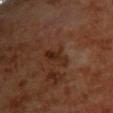Q: Is there a histopathology result?
A: no biopsy performed (imaged during a skin exam)
Q: What is the anatomic site?
A: the back
Q: What lighting was used for the tile?
A: cross-polarized illumination
Q: Who is the patient?
A: female
Q: How was this image acquired?
A: 15 mm crop, total-body photography
Q: What is the lesion's diameter?
A: about 3.5 mm